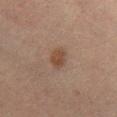follow-up — catalogued during a skin exam; not biopsied | image-analysis metrics — an area of roughly 4.5 mm², an outline eccentricity of about 0.65 (0 = round, 1 = elongated), and a symmetry-axis asymmetry near 0.2; a border-irregularity index near 1.5/10, a within-lesion color-variation index near 2/10, and a peripheral color-asymmetry measure near 0.5 | location — the left lower leg | image source — 15 mm crop, total-body photography | subject — female, aged around 55.